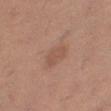<record>
  <biopsy_status>not biopsied; imaged during a skin examination</biopsy_status>
</record>